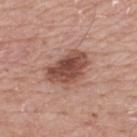Assessment:
The lesion was photographed on a routine skin check and not biopsied; there is no pathology result.
Image and clinical context:
On the mid back. A 15 mm close-up tile from a total-body photography series done for melanoma screening. A male patient aged 73 to 77. About 5 mm across. The tile uses white-light illumination.